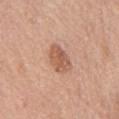Context:
Located on the chest. A close-up tile cropped from a whole-body skin photograph, about 15 mm across. This is a white-light tile. Automated image analysis of the tile measured a border-irregularity index near 2/10 and a within-lesion color-variation index near 3.5/10. The software also gave a detector confidence of about 100 out of 100 that the crop contains a lesion. A male subject, aged around 75.